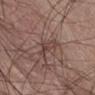<case>
<biopsy_status>not biopsied; imaged during a skin examination</biopsy_status>
<automated_metrics>
  <cielab_L>42</cielab_L>
  <cielab_a>17</cielab_a>
  <cielab_b>21</cielab_b>
  <vs_skin_darker_L>7.0</vs_skin_darker_L>
  <vs_skin_contrast_norm>6.0</vs_skin_contrast_norm>
  <nevus_likeness_0_100>0</nevus_likeness_0_100>
</automated_metrics>
<site>abdomen</site>
<patient>
  <sex>male</sex>
  <age_approx>75</age_approx>
</patient>
<lighting>white-light</lighting>
<image>
  <source>total-body photography crop</source>
  <field_of_view_mm>15</field_of_view_mm>
</image>
</case>